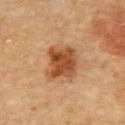notes = no biopsy performed (imaged during a skin exam) | subject = male, aged approximately 85 or older | illumination = cross-polarized | anatomic site = the upper back | image source = 15 mm crop, total-body photography | lesion size = ~4 mm (longest diameter).